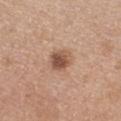Q: Is there a histopathology result?
A: no biopsy performed (imaged during a skin exam)
Q: Illumination type?
A: white-light
Q: What is the anatomic site?
A: the chest
Q: Patient demographics?
A: female, about 30 years old
Q: How was this image acquired?
A: ~15 mm crop, total-body skin-cancer survey